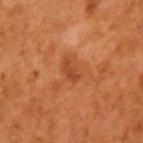Notes:
- notes: total-body-photography surveillance lesion; no biopsy
- site: the left upper arm
- illumination: cross-polarized illumination
- image-analysis metrics: an automated nevus-likeness rating near 0 out of 100 and lesion-presence confidence of about 100/100
- subject: male, aged 58 to 62
- imaging modality: total-body-photography crop, ~15 mm field of view
- size: ≈2.5 mm